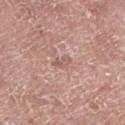This lesion was catalogued during total-body skin photography and was not selected for biopsy.
The lesion is on the left lower leg.
A roughly 15 mm field-of-view crop from a total-body skin photograph.
The subject is a male aged around 75.